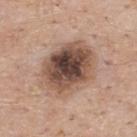The lesion was photographed on a routine skin check and not biopsied; there is no pathology result.
The subject is a male roughly 60 years of age.
The lesion is on the back.
This image is a 15 mm lesion crop taken from a total-body photograph.
Measured at roughly 6.5 mm in maximum diameter.
Imaged with white-light lighting.
The lesion-visualizer software estimated an eccentricity of roughly 0.65 and a shape-asymmetry score of about 0.15 (0 = symmetric). The software also gave a classifier nevus-likeness of about 10/100.